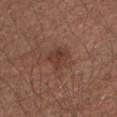{"biopsy_status": "not biopsied; imaged during a skin examination", "site": "right upper arm", "automated_metrics": {"border_irregularity_0_10": 3.0, "color_variation_0_10": 2.0}, "image": {"source": "total-body photography crop", "field_of_view_mm": 15}, "patient": {"sex": "male", "age_approx": 55}, "lighting": "white-light"}